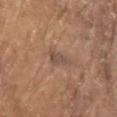<record>
  <biopsy_status>not biopsied; imaged during a skin examination</biopsy_status>
  <site>arm</site>
  <patient>
    <sex>male</sex>
    <age_approx>80</age_approx>
  </patient>
  <image>
    <source>total-body photography crop</source>
    <field_of_view_mm>15</field_of_view_mm>
  </image>
</record>